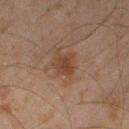Part of a total-body skin-imaging series; this lesion was reviewed on a skin check and was not flagged for biopsy. Automated image analysis of the tile measured a color-variation rating of about 2.5/10 and a peripheral color-asymmetry measure near 1. The analysis additionally found a classifier nevus-likeness of about 40/100. Cropped from a whole-body photographic skin survey; the tile spans about 15 mm. The tile uses cross-polarized illumination. On the right thigh. A male patient aged 43 to 47.